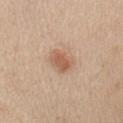acquisition = total-body-photography crop, ~15 mm field of view; subject = female, in their mid- to late 50s; illumination = white-light illumination; anatomic site = the arm.